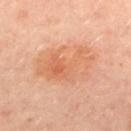Q: Was a biopsy performed?
A: no biopsy performed (imaged during a skin exam)
Q: What is the anatomic site?
A: the chest
Q: What kind of image is this?
A: 15 mm crop, total-body photography
Q: Lesion size?
A: ~7.5 mm (longest diameter)
Q: Patient demographics?
A: female, aged 48–52